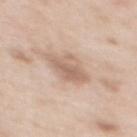Recorded during total-body skin imaging; not selected for excision or biopsy. Imaged with white-light lighting. The recorded lesion diameter is about 4 mm. A female subject aged 48 to 52. This image is a 15 mm lesion crop taken from a total-body photograph. Located on the upper back.